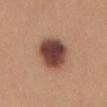| key | value |
|---|---|
| notes | imaged on a skin check; not biopsied |
| lighting | white-light illumination |
| lesion diameter | ≈5 mm |
| patient | female, aged around 25 |
| image source | 15 mm crop, total-body photography |
| anatomic site | the abdomen |